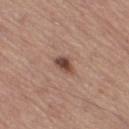Q: Was a biopsy performed?
A: catalogued during a skin exam; not biopsied
Q: Lesion location?
A: the right thigh
Q: Who is the patient?
A: female, aged approximately 65
Q: What is the imaging modality?
A: 15 mm crop, total-body photography
Q: Illumination type?
A: white-light illumination
Q: How large is the lesion?
A: ~2.5 mm (longest diameter)